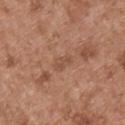Notes:
– biopsy status — catalogued during a skin exam; not biopsied
– illumination — white-light
– image — 15 mm crop, total-body photography
– site — the upper back
– patient — male, aged around 55
– automated metrics — a lesion color around L≈50 a*≈21 b*≈30 in CIELAB and a lesion–skin lightness drop of about 7; internal color variation of about 0 on a 0–10 scale and a peripheral color-asymmetry measure near 0